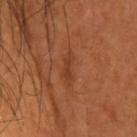Part of a total-body skin-imaging series; this lesion was reviewed on a skin check and was not flagged for biopsy. The lesion is on the head or neck. The lesion's longest dimension is about 3 mm. A male subject aged 38 to 42. The lesion-visualizer software estimated a border-irregularity rating of about 4/10 and a peripheral color-asymmetry measure near 0. It also reported a lesion-detection confidence of about 95/100. A close-up tile cropped from a whole-body skin photograph, about 15 mm across. Captured under cross-polarized illumination.